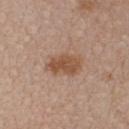The lesion was tiled from a total-body skin photograph and was not biopsied. Imaged with white-light lighting. The patient is a female approximately 35 years of age. A 15 mm close-up tile from a total-body photography series done for melanoma screening. From the chest.